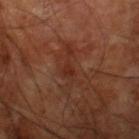Captured during whole-body skin photography for melanoma surveillance; the lesion was not biopsied. Cropped from a whole-body photographic skin survey; the tile spans about 15 mm. Imaged with cross-polarized lighting. The subject is a male approximately 60 years of age. The lesion is on the right lower leg. About 5 mm across.